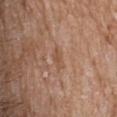Assessment:
This lesion was catalogued during total-body skin photography and was not selected for biopsy.
Acquisition and patient details:
The subject is a female aged 83 or over. A region of skin cropped from a whole-body photographic capture, roughly 15 mm wide. The lesion is on the upper back. Imaged with white-light lighting.